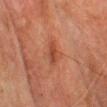Q: Is there a histopathology result?
A: catalogued during a skin exam; not biopsied
Q: What did automated image analysis measure?
A: a lesion area of about 5 mm² and an eccentricity of roughly 0.7; a mean CIELAB color near L≈39 a*≈24 b*≈30, a lesion–skin lightness drop of about 7, and a normalized border contrast of about 6.5; a classifier nevus-likeness of about 15/100 and a lesion-detection confidence of about 100/100
Q: How was this image acquired?
A: total-body-photography crop, ~15 mm field of view
Q: Where on the body is the lesion?
A: the front of the torso
Q: Who is the patient?
A: male, about 75 years old
Q: What is the lesion's diameter?
A: about 2.5 mm
Q: What lighting was used for the tile?
A: cross-polarized illumination